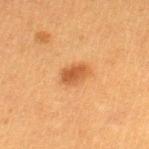Impression: The lesion was photographed on a routine skin check and not biopsied; there is no pathology result. Background: A female subject about 40 years old. Longest diameter approximately 3 mm. This image is a 15 mm lesion crop taken from a total-body photograph. Located on the leg. The tile uses cross-polarized illumination.